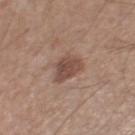<tbp_lesion>
<biopsy_status>not biopsied; imaged during a skin examination</biopsy_status>
<lighting>white-light</lighting>
<automated_metrics>
  <border_irregularity_0_10>3.0</border_irregularity_0_10>
  <peripheral_color_asymmetry>1.0</peripheral_color_asymmetry>
  <lesion_detection_confidence_0_100>100</lesion_detection_confidence_0_100>
</automated_metrics>
<site>right thigh</site>
<image>
  <source>total-body photography crop</source>
  <field_of_view_mm>15</field_of_view_mm>
</image>
<patient>
  <sex>male</sex>
  <age_approx>65</age_approx>
</patient>
<lesion_size>
  <long_diameter_mm_approx>3.5</long_diameter_mm_approx>
</lesion_size>
</tbp_lesion>